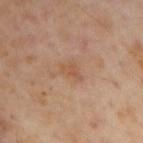Q: Is there a histopathology result?
A: imaged on a skin check; not biopsied
Q: How was the tile lit?
A: cross-polarized
Q: Lesion location?
A: the right upper arm
Q: What did automated image analysis measure?
A: an area of roughly 4 mm² and a shape-asymmetry score of about 0.3 (0 = symmetric); an average lesion color of about L≈54 a*≈20 b*≈32 (CIELAB) and a lesion-to-skin contrast of about 5 (normalized; higher = more distinct)
Q: Lesion size?
A: ~3 mm (longest diameter)
Q: What are the patient's age and sex?
A: male, aged 53–57
Q: How was this image acquired?
A: total-body-photography crop, ~15 mm field of view